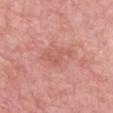| feature | finding |
|---|---|
| workup | catalogued during a skin exam; not biopsied |
| lighting | white-light |
| TBP lesion metrics | a lesion color around L≈58 a*≈29 b*≈28 in CIELAB, a lesion–skin lightness drop of about 7, and a normalized border contrast of about 4.5; a border-irregularity rating of about 7.5/10, a color-variation rating of about 2/10, and radial color variation of about 0.5; an automated nevus-likeness rating near 0 out of 100 and lesion-presence confidence of about 100/100 |
| acquisition | ~15 mm tile from a whole-body skin photo |
| location | the back |
| subject | female, aged around 65 |
| size | about 4 mm |